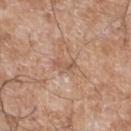Recorded during total-body skin imaging; not selected for excision or biopsy. The subject is a male in their 60s. The tile uses white-light illumination. A lesion tile, about 15 mm wide, cut from a 3D total-body photograph. The lesion is on the left lower leg. The recorded lesion diameter is about 3 mm.